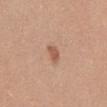No biopsy was performed on this lesion — it was imaged during a full skin examination and was not determined to be concerning. A roughly 15 mm field-of-view crop from a total-body skin photograph. The subject is a female in their mid-30s. From the mid back.